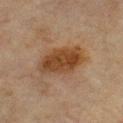follow-up=catalogued during a skin exam; not biopsied | illumination=cross-polarized | lesion diameter=≈5.5 mm | image=~15 mm crop, total-body skin-cancer survey | TBP lesion metrics=a footprint of about 14 mm² and a shape eccentricity near 0.85; a mean CIELAB color near L≈33 a*≈17 b*≈28, roughly 10 lightness units darker than nearby skin, and a normalized lesion–skin contrast near 10; border irregularity of about 2 on a 0–10 scale, a color-variation rating of about 3.5/10, and radial color variation of about 1 | body site=the upper back | subject=male, approximately 65 years of age.